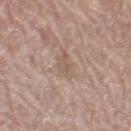The lesion was photographed on a routine skin check and not biopsied; there is no pathology result. On the right thigh. The recorded lesion diameter is about 3.5 mm. This is a white-light tile. A lesion tile, about 15 mm wide, cut from a 3D total-body photograph. The subject is a female in their 60s.